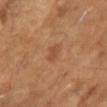The lesion was photographed on a routine skin check and not biopsied; there is no pathology result. A close-up tile cropped from a whole-body skin photograph, about 15 mm across. A male patient, aged approximately 65.